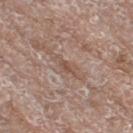biopsy_status: not biopsied; imaged during a skin examination
image:
  source: total-body photography crop
  field_of_view_mm: 15
patient:
  sex: female
  age_approx: 75
site: right thigh